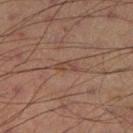Captured during whole-body skin photography for melanoma surveillance; the lesion was not biopsied. The lesion is located on the left thigh. The lesion's longest dimension is about 2.5 mm. Automated image analysis of the tile measured a footprint of about 2.5 mm². And it measured a nevus-likeness score of about 0/100 and lesion-presence confidence of about 60/100. A region of skin cropped from a whole-body photographic capture, roughly 15 mm wide. A male subject aged 68 to 72. Imaged with cross-polarized lighting.